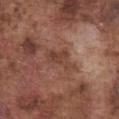follow-up: imaged on a skin check; not biopsied | image: total-body-photography crop, ~15 mm field of view | body site: the chest | illumination: white-light | lesion size: ≈4.5 mm | subject: male, in their mid- to late 70s | image-analysis metrics: a border-irregularity rating of about 6/10, internal color variation of about 2.5 on a 0–10 scale, and peripheral color asymmetry of about 1.